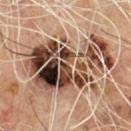The lesion was photographed on a routine skin check and not biopsied; there is no pathology result. Located on the upper back. A male subject about 60 years old. Captured under cross-polarized illumination. The lesion's longest dimension is about 10.5 mm. Cropped from a total-body skin-imaging series; the visible field is about 15 mm.